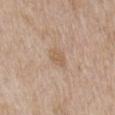The lesion was tiled from a total-body skin photograph and was not biopsied. The lesion is located on the mid back. The lesion's longest dimension is about 3 mm. The subject is a female in their 60s. A lesion tile, about 15 mm wide, cut from a 3D total-body photograph.